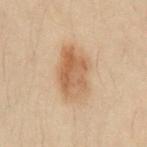The lesion was tiled from a total-body skin photograph and was not biopsied.
Imaged with cross-polarized lighting.
The total-body-photography lesion software estimated an area of roughly 16 mm², an outline eccentricity of about 0.8 (0 = round, 1 = elongated), and a shape-asymmetry score of about 0.25 (0 = symmetric). The analysis additionally found a classifier nevus-likeness of about 95/100 and a detector confidence of about 100 out of 100 that the crop contains a lesion.
The subject is a male aged around 30.
A roughly 15 mm field-of-view crop from a total-body skin photograph.
Located on the abdomen.